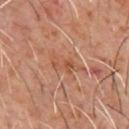notes: catalogued during a skin exam; not biopsied
image source: ~15 mm crop, total-body skin-cancer survey
site: the chest
image-analysis metrics: a footprint of about 6.5 mm², an eccentricity of roughly 0.9, and a shape-asymmetry score of about 0.35 (0 = symmetric); a border-irregularity index near 5/10, a color-variation rating of about 5/10, and radial color variation of about 2
lesion size: ≈4.5 mm
subject: male, roughly 60 years of age